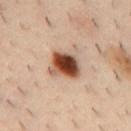notes: no biopsy performed (imaged during a skin exam) | illumination: cross-polarized illumination | automated lesion analysis: a lesion area of about 10 mm², an eccentricity of roughly 0.6, and two-axis asymmetry of about 0.25; a border-irregularity rating of about 2.5/10 and a peripheral color-asymmetry measure near 3.5 | patient: male, aged 38–42 | diameter: ≈3.5 mm | image source: total-body-photography crop, ~15 mm field of view | anatomic site: the mid back.